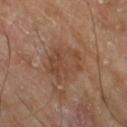This lesion was catalogued during total-body skin photography and was not selected for biopsy. The lesion is on the leg. A 15 mm crop from a total-body photograph taken for skin-cancer surveillance. Longest diameter approximately 4.5 mm. The patient is a male in their mid- to late 60s. This is a cross-polarized tile. The lesion-visualizer software estimated a shape eccentricity near 0.6 and a shape-asymmetry score of about 0.25 (0 = symmetric). It also reported an automated nevus-likeness rating near 0 out of 100 and lesion-presence confidence of about 100/100.